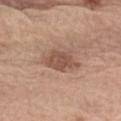Impression: Part of a total-body skin-imaging series; this lesion was reviewed on a skin check and was not flagged for biopsy. Acquisition and patient details: A 15 mm crop from a total-body photograph taken for skin-cancer surveillance. The lesion is located on the left upper arm. A male subject approximately 70 years of age.